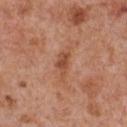Assessment: The lesion was photographed on a routine skin check and not biopsied; there is no pathology result. Image and clinical context: A 15 mm crop from a total-body photograph taken for skin-cancer surveillance. An algorithmic analysis of the crop reported a mean CIELAB color near L≈50 a*≈25 b*≈34, a lesion–skin lightness drop of about 9, and a normalized border contrast of about 7. Imaged with white-light lighting. The subject is a male aged 63–67. The recorded lesion diameter is about 3.5 mm. The lesion is on the chest.